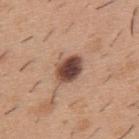This lesion was catalogued during total-body skin photography and was not selected for biopsy. Automated tile analysis of the lesion measured a border-irregularity index near 1.5/10, a within-lesion color-variation index near 5.5/10, and a peripheral color-asymmetry measure near 1.5. This is a white-light tile. The lesion's longest dimension is about 3.5 mm. A male patient aged 38 to 42. The lesion is on the upper back. This image is a 15 mm lesion crop taken from a total-body photograph.